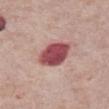<record>
<biopsy_status>not biopsied; imaged during a skin examination</biopsy_status>
<image>
  <source>total-body photography crop</source>
  <field_of_view_mm>15</field_of_view_mm>
</image>
<patient>
  <sex>male</sex>
  <age_approx>75</age_approx>
</patient>
<lesion_size>
  <long_diameter_mm_approx>3.5</long_diameter_mm_approx>
</lesion_size>
<site>front of the torso</site>
<lighting>white-light</lighting>
</record>